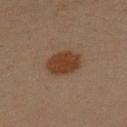notes = no biopsy performed (imaged during a skin exam); size = ≈4.5 mm; subject = female, about 40 years old; tile lighting = cross-polarized; location = the left upper arm; acquisition = ~15 mm tile from a whole-body skin photo.